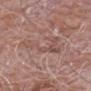No biopsy was performed on this lesion — it was imaged during a full skin examination and was not determined to be concerning.
Automated image analysis of the tile measured an area of roughly 12 mm² and an outline eccentricity of about 0.85 (0 = round, 1 = elongated). The software also gave an average lesion color of about L≈52 a*≈19 b*≈23 (CIELAB), about 6 CIELAB-L* units darker than the surrounding skin, and a lesion-to-skin contrast of about 4.5 (normalized; higher = more distinct). The software also gave lesion-presence confidence of about 65/100.
A region of skin cropped from a whole-body photographic capture, roughly 15 mm wide.
A male patient, aged around 75.
Located on the right forearm.
The tile uses white-light illumination.
Measured at roughly 6.5 mm in maximum diameter.